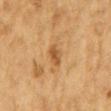Impression: Part of a total-body skin-imaging series; this lesion was reviewed on a skin check and was not flagged for biopsy. Acquisition and patient details: The tile uses cross-polarized illumination. The lesion is located on the mid back. A 15 mm close-up tile from a total-body photography series done for melanoma screening. About 3 mm across. A male subject aged around 85 or older.